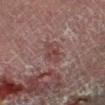This lesion was catalogued during total-body skin photography and was not selected for biopsy. The lesion's longest dimension is about 3.5 mm. Located on the left lower leg. This is a cross-polarized tile. A region of skin cropped from a whole-body photographic capture, roughly 15 mm wide. A male subject about 55 years old.